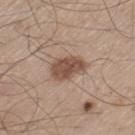A male patient approximately 60 years of age.
On the left thigh.
A 15 mm close-up tile from a total-body photography series done for melanoma screening.